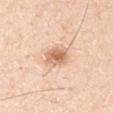This lesion was catalogued during total-body skin photography and was not selected for biopsy.
A lesion tile, about 15 mm wide, cut from a 3D total-body photograph.
Measured at roughly 3.5 mm in maximum diameter.
A male patient approximately 30 years of age.
The lesion is located on the right upper arm.
Imaged with white-light lighting.